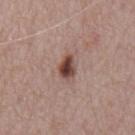Captured during whole-body skin photography for melanoma surveillance; the lesion was not biopsied.
Located on the back.
A 15 mm crop from a total-body photograph taken for skin-cancer surveillance.
A male patient approximately 75 years of age.